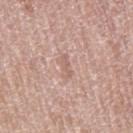follow-up — total-body-photography surveillance lesion; no biopsy | imaging modality — ~15 mm tile from a whole-body skin photo | diameter — ~3 mm (longest diameter) | patient — female, aged 58 to 62 | tile lighting — white-light | site — the right thigh | TBP lesion metrics — a lesion-to-skin contrast of about 5 (normalized; higher = more distinct); a border-irregularity rating of about 4.5/10; a nevus-likeness score of about 0/100.